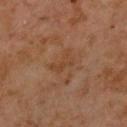  site: chest
  patient:
    sex: male
    age_approx: 60
  lighting: cross-polarized
  image:
    source: total-body photography crop
    field_of_view_mm: 15